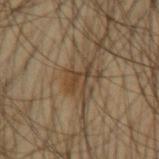Q: Is there a histopathology result?
A: no biopsy performed (imaged during a skin exam)
Q: Automated lesion metrics?
A: a lesion area of about 4 mm², an eccentricity of roughly 0.8, and a shape-asymmetry score of about 0.6 (0 = symmetric); an average lesion color of about L≈39 a*≈14 b*≈29 (CIELAB), a lesion–skin lightness drop of about 8, and a normalized lesion–skin contrast near 7.5; a border-irregularity rating of about 9.5/10, internal color variation of about 0 on a 0–10 scale, and radial color variation of about 0; an automated nevus-likeness rating near 0 out of 100 and lesion-presence confidence of about 55/100
Q: What lighting was used for the tile?
A: cross-polarized
Q: Where on the body is the lesion?
A: the arm
Q: How large is the lesion?
A: ~3.5 mm (longest diameter)
Q: What is the imaging modality?
A: ~15 mm tile from a whole-body skin photo
Q: Who is the patient?
A: male, roughly 45 years of age